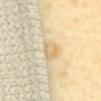This is a cross-polarized tile.
The lesion is located on the chest.
A female subject, aged around 55.
The total-body-photography lesion software estimated a footprint of about 6 mm², an outline eccentricity of about 0.75 (0 = round, 1 = elongated), and two-axis asymmetry of about 0.25. The analysis additionally found a classifier nevus-likeness of about 0/100 and lesion-presence confidence of about 95/100.
The lesion's longest dimension is about 3.5 mm.
A region of skin cropped from a whole-body photographic capture, roughly 15 mm wide.